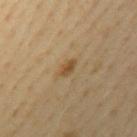The lesion was photographed on a routine skin check and not biopsied; there is no pathology result.
On the arm.
A male patient about 40 years old.
The lesion's longest dimension is about 2.5 mm.
This image is a 15 mm lesion crop taken from a total-body photograph.
The lesion-visualizer software estimated a mean CIELAB color near L≈49 a*≈15 b*≈37, roughly 9 lightness units darker than nearby skin, and a lesion-to-skin contrast of about 8 (normalized; higher = more distinct).
Captured under cross-polarized illumination.